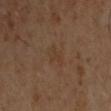Case summary:
- follow-up · total-body-photography surveillance lesion; no biopsy
- automated metrics · a mean CIELAB color near L≈36 a*≈16 b*≈28 and a lesion–skin lightness drop of about 4; a color-variation rating of about 0.5/10 and a peripheral color-asymmetry measure near 0; a nevus-likeness score of about 0/100 and a detector confidence of about 100 out of 100 that the crop contains a lesion
- acquisition · ~15 mm crop, total-body skin-cancer survey
- location · the chest
- lesion size · ≈3 mm
- tile lighting · cross-polarized
- subject · male, approximately 45 years of age